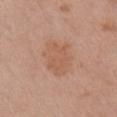Impression:
Imaged during a routine full-body skin examination; the lesion was not biopsied and no histopathology is available.
Image and clinical context:
On the front of the torso. A female subject in their mid-60s. Cropped from a whole-body photographic skin survey; the tile spans about 15 mm.